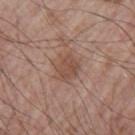  biopsy_status: not biopsied; imaged during a skin examination
  patient:
    sex: male
    age_approx: 65
  site: left upper arm
  lighting: white-light
  automated_metrics:
    shape_asymmetry: 0.2
    cielab_L: 49
    cielab_a: 19
    cielab_b: 27
    vs_skin_contrast_norm: 6.5
    color_variation_0_10: 2.0
    nevus_likeness_0_100: 15
  image:
    source: total-body photography crop
    field_of_view_mm: 15